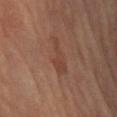{
  "biopsy_status": "not biopsied; imaged during a skin examination",
  "automated_metrics": {
    "cielab_L": 40,
    "cielab_a": 21,
    "cielab_b": 26,
    "vs_skin_contrast_norm": 5.0
  },
  "lesion_size": {
    "long_diameter_mm_approx": 4.5
  },
  "site": "left arm",
  "patient": {
    "sex": "female",
    "age_approx": 65
  },
  "lighting": "cross-polarized",
  "image": {
    "source": "total-body photography crop",
    "field_of_view_mm": 15
  }
}